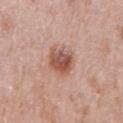Clinical impression:
This lesion was catalogued during total-body skin photography and was not selected for biopsy.
Clinical summary:
A female patient aged 38 to 42. Automated image analysis of the tile measured a border-irregularity rating of about 1.5/10 and a peripheral color-asymmetry measure near 2. A 15 mm close-up tile from a total-body photography series done for melanoma screening. Approximately 3.5 mm at its widest. On the right forearm. The tile uses white-light illumination.